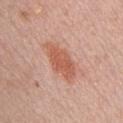Part of a total-body skin-imaging series; this lesion was reviewed on a skin check and was not flagged for biopsy.
Measured at roughly 5.5 mm in maximum diameter.
The tile uses white-light illumination.
A roughly 15 mm field-of-view crop from a total-body skin photograph.
The lesion is on the front of the torso.
A male patient, aged approximately 30.
Automated tile analysis of the lesion measured an eccentricity of roughly 0.9. It also reported an average lesion color of about L≈58 a*≈26 b*≈32 (CIELAB), a lesion–skin lightness drop of about 10, and a normalized lesion–skin contrast near 7.5. The software also gave a border-irregularity rating of about 3/10 and a color-variation rating of about 2.5/10.